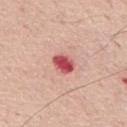{"biopsy_status": "not biopsied; imaged during a skin examination", "site": "mid back", "lighting": "white-light", "patient": {"sex": "male", "age_approx": 75}, "image": {"source": "total-body photography crop", "field_of_view_mm": 15}}